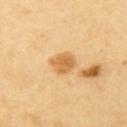  biopsy_status: not biopsied; imaged during a skin examination
  patient:
    sex: male
    age_approx: 60
  site: arm
  image:
    source: total-body photography crop
    field_of_view_mm: 15